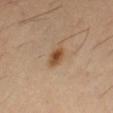Assessment:
Imaged during a routine full-body skin examination; the lesion was not biopsied and no histopathology is available.
Background:
On the right thigh. A lesion tile, about 15 mm wide, cut from a 3D total-body photograph. A male subject, aged 58–62. The recorded lesion diameter is about 3 mm. The tile uses cross-polarized illumination.